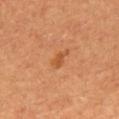follow-up = total-body-photography surveillance lesion; no biopsy | size = about 3 mm | image = ~15 mm tile from a whole-body skin photo | body site = the back | patient = female, in their mid-60s.